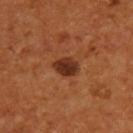Assessment:
This lesion was catalogued during total-body skin photography and was not selected for biopsy.
Background:
A 15 mm close-up tile from a total-body photography series done for melanoma screening. A male patient, in their mid- to late 50s. Located on the upper back.